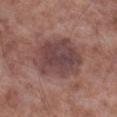Q: Was a biopsy performed?
A: total-body-photography surveillance lesion; no biopsy
Q: How was this image acquired?
A: total-body-photography crop, ~15 mm field of view
Q: What lighting was used for the tile?
A: white-light illumination
Q: Patient demographics?
A: male, aged approximately 55
Q: Where on the body is the lesion?
A: the right lower leg
Q: What is the lesion's diameter?
A: about 6.5 mm